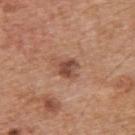location: the upper back | imaging modality: ~15 mm tile from a whole-body skin photo | diameter: ~3 mm (longest diameter) | subject: male, about 65 years old.